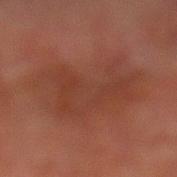Q: Was a biopsy performed?
A: catalogued during a skin exam; not biopsied
Q: Automated lesion metrics?
A: two-axis asymmetry of about 0.3; border irregularity of about 4.5 on a 0–10 scale and peripheral color asymmetry of about 1; a classifier nevus-likeness of about 0/100 and a lesion-detection confidence of about 100/100
Q: What is the lesion's diameter?
A: ≈9 mm
Q: What is the imaging modality?
A: ~15 mm tile from a whole-body skin photo
Q: Where on the body is the lesion?
A: the right lower leg
Q: Patient demographics?
A: male, aged 68 to 72